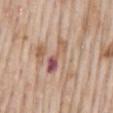From the mid back. A male subject, roughly 65 years of age. The tile uses white-light illumination. Measured at roughly 4.5 mm in maximum diameter. A 15 mm crop from a total-body photograph taken for skin-cancer surveillance.